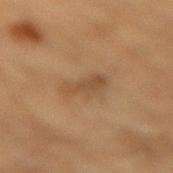{
  "biopsy_status": "not biopsied; imaged during a skin examination",
  "image": {
    "source": "total-body photography crop",
    "field_of_view_mm": 15
  },
  "lighting": "cross-polarized",
  "site": "mid back",
  "patient": {
    "sex": "male",
    "age_approx": "85+"
  }
}